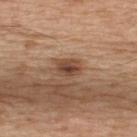Q: What lighting was used for the tile?
A: white-light illumination
Q: What is the imaging modality?
A: ~15 mm tile from a whole-body skin photo
Q: What is the anatomic site?
A: the upper back
Q: What are the patient's age and sex?
A: male, in their mid-60s